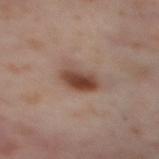follow-up — catalogued during a skin exam; not biopsied | acquisition — total-body-photography crop, ~15 mm field of view | illumination — cross-polarized illumination | subject — female, about 55 years old | TBP lesion metrics — an area of roughly 8 mm², a shape eccentricity near 0.8, and a symmetry-axis asymmetry near 0.25 | lesion diameter — ≈4 mm | site — the right thigh.